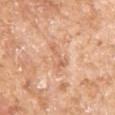location = the left upper arm
image source = ~15 mm tile from a whole-body skin photo
subject = female, about 75 years old
diameter = ≈4 mm